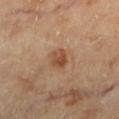workup = no biopsy performed (imaged during a skin exam) | acquisition = ~15 mm crop, total-body skin-cancer survey | anatomic site = the left lower leg | lesion diameter = about 3 mm | tile lighting = cross-polarized illumination | patient = female, aged approximately 60.